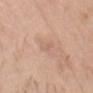* biopsy status — no biopsy performed (imaged during a skin exam)
* illumination — white-light
* image source — ~15 mm crop, total-body skin-cancer survey
* anatomic site — the head or neck
* lesion diameter — ~1.5 mm (longest diameter)
* patient — male, in their 30s